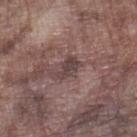Case summary:
• workup · total-body-photography surveillance lesion; no biopsy
• image-analysis metrics · a border-irregularity rating of about 4/10, a within-lesion color-variation index near 1.5/10, and peripheral color asymmetry of about 0.5; a classifier nevus-likeness of about 0/100 and a lesion-detection confidence of about 95/100
• lesion diameter · ~3 mm (longest diameter)
• subject · male, in their mid- to late 70s
• acquisition · ~15 mm tile from a whole-body skin photo
• site · the left lower leg
• illumination · white-light